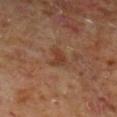No biopsy was performed on this lesion — it was imaged during a full skin examination and was not determined to be concerning. On the right lower leg. A region of skin cropped from a whole-body photographic capture, roughly 15 mm wide. The subject is a male aged approximately 60. About 3 mm across. An algorithmic analysis of the crop reported a border-irregularity rating of about 4.5/10, internal color variation of about 2 on a 0–10 scale, and a peripheral color-asymmetry measure near 1.